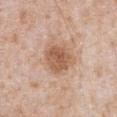patient: male, aged 63 to 67
lesion size: about 4 mm
acquisition: 15 mm crop, total-body photography
location: the abdomen
illumination: white-light illumination